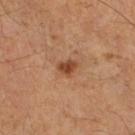– notes: imaged on a skin check; not biopsied
– TBP lesion metrics: an outline eccentricity of about 0.65 (0 = round, 1 = elongated) and a shape-asymmetry score of about 0.3 (0 = symmetric); a classifier nevus-likeness of about 85/100
– size: about 2.5 mm
– anatomic site: the right lower leg
– patient: male, aged around 55
– image: total-body-photography crop, ~15 mm field of view